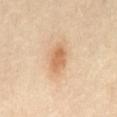Impression: The lesion was photographed on a routine skin check and not biopsied; there is no pathology result. Context: The lesion-visualizer software estimated a lesion area of about 6.5 mm² and an eccentricity of roughly 0.8. And it measured a lesion–skin lightness drop of about 11 and a normalized border contrast of about 7. It also reported an automated nevus-likeness rating near 95 out of 100 and a detector confidence of about 100 out of 100 that the crop contains a lesion. A male patient, in their mid- to late 60s. From the abdomen. The recorded lesion diameter is about 3.5 mm. This image is a 15 mm lesion crop taken from a total-body photograph.